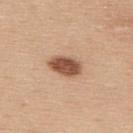Q: Was a biopsy performed?
A: catalogued during a skin exam; not biopsied
Q: How large is the lesion?
A: ≈4 mm
Q: What are the patient's age and sex?
A: male, about 35 years old
Q: Where on the body is the lesion?
A: the upper back
Q: What kind of image is this?
A: ~15 mm tile from a whole-body skin photo